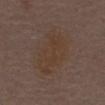follow-up: catalogued during a skin exam; not biopsied
image-analysis metrics: an area of roughly 19 mm², an eccentricity of roughly 0.85, and a shape-asymmetry score of about 0.25 (0 = symmetric); a border-irregularity rating of about 3.5/10 and a peripheral color-asymmetry measure near 0.5; lesion-presence confidence of about 100/100
imaging modality: 15 mm crop, total-body photography
subject: male, roughly 70 years of age
site: the abdomen
tile lighting: white-light illumination
diameter: about 7 mm